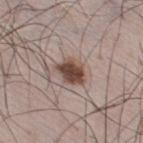workup: catalogued during a skin exam; not biopsied
subject: male, aged 63 to 67
automated metrics: about 15 CIELAB-L* units darker than the surrounding skin; an automated nevus-likeness rating near 95 out of 100 and a detector confidence of about 100 out of 100 that the crop contains a lesion
tile lighting: white-light illumination
location: the right thigh
image: ~15 mm crop, total-body skin-cancer survey
lesion diameter: ~3.5 mm (longest diameter)